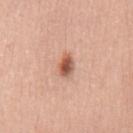Findings:
• follow-up — no biopsy performed (imaged during a skin exam)
• body site — the mid back
• illumination — white-light illumination
• lesion diameter — about 3 mm
• TBP lesion metrics — an area of roughly 4.5 mm², a shape eccentricity near 0.8, and a shape-asymmetry score of about 0.25 (0 = symmetric); a lesion color around L≈57 a*≈24 b*≈31 in CIELAB, roughly 14 lightness units darker than nearby skin, and a normalized lesion–skin contrast near 9
• acquisition — ~15 mm tile from a whole-body skin photo
• subject — female, roughly 40 years of age